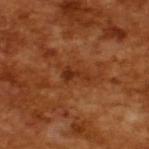Assessment: Captured during whole-body skin photography for melanoma surveillance; the lesion was not biopsied. Clinical summary: Captured under cross-polarized illumination. A male patient approximately 65 years of age. Approximately 3 mm at its widest. A lesion tile, about 15 mm wide, cut from a 3D total-body photograph.